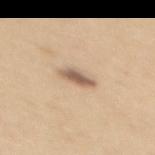Recorded during total-body skin imaging; not selected for excision or biopsy.
A 15 mm close-up extracted from a 3D total-body photography capture.
An algorithmic analysis of the crop reported an average lesion color of about L≈60 a*≈16 b*≈30 (CIELAB), a lesion–skin lightness drop of about 14, and a normalized lesion–skin contrast near 9. And it measured internal color variation of about 3 on a 0–10 scale and a peripheral color-asymmetry measure near 1. It also reported an automated nevus-likeness rating near 95 out of 100 and a lesion-detection confidence of about 100/100.
A female patient aged 28–32.
On the mid back.